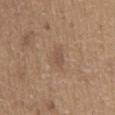Imaged during a routine full-body skin examination; the lesion was not biopsied and no histopathology is available. A male subject, roughly 65 years of age. This image is a 15 mm lesion crop taken from a total-body photograph. The lesion is located on the abdomen.